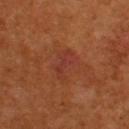Captured during whole-body skin photography for melanoma surveillance; the lesion was not biopsied. A roughly 15 mm field-of-view crop from a total-body skin photograph. The subject is a male aged 53–57. The lesion is located on the upper back. The lesion's longest dimension is about 4 mm. Captured under cross-polarized illumination.